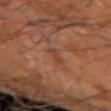{
  "biopsy_status": "not biopsied; imaged during a skin examination",
  "image": {
    "source": "total-body photography crop",
    "field_of_view_mm": 15
  },
  "automated_metrics": {
    "cielab_L": 42,
    "cielab_a": 24,
    "cielab_b": 29,
    "vs_skin_darker_L": 6.0,
    "vs_skin_contrast_norm": 5.5
  },
  "patient": {
    "age_approx": 65
  },
  "site": "left forearm",
  "lesion_size": {
    "long_diameter_mm_approx": 2.5
  }
}